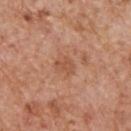follow-up: imaged on a skin check; not biopsied
body site: the upper back
image-analysis metrics: an area of roughly 3.5 mm², an outline eccentricity of about 0.75 (0 = round, 1 = elongated), and two-axis asymmetry of about 0.35; a mean CIELAB color near L≈53 a*≈24 b*≈33 and a lesion–skin lightness drop of about 7; a border-irregularity index near 3.5/10, internal color variation of about 1 on a 0–10 scale, and radial color variation of about 0.5; a nevus-likeness score of about 0/100 and a detector confidence of about 100 out of 100 that the crop contains a lesion
image: ~15 mm crop, total-body skin-cancer survey
size: ≈2.5 mm
patient: male, aged approximately 65